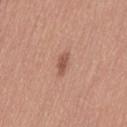Clinical impression:
Part of a total-body skin-imaging series; this lesion was reviewed on a skin check and was not flagged for biopsy.
Background:
About 3 mm across. A female subject, approximately 40 years of age. Located on the left thigh. Cropped from a total-body skin-imaging series; the visible field is about 15 mm.